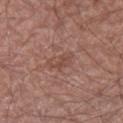Assessment:
Recorded during total-body skin imaging; not selected for excision or biopsy.
Context:
Cropped from a whole-body photographic skin survey; the tile spans about 15 mm. Longest diameter approximately 3.5 mm. Automated image analysis of the tile measured a classifier nevus-likeness of about 0/100 and a lesion-detection confidence of about 100/100. The patient is a male about 60 years old. On the right lower leg. Imaged with white-light lighting.